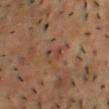biopsy status: catalogued during a skin exam; not biopsied
image source: total-body-photography crop, ~15 mm field of view
location: the chest
subject: male, aged approximately 55
lesion diameter: ~2.5 mm (longest diameter)
illumination: cross-polarized